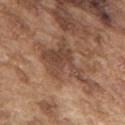The lesion was tiled from a total-body skin photograph and was not biopsied. The tile uses white-light illumination. From the back. The patient is a male roughly 75 years of age. Automated image analysis of the tile measured a lesion color around L≈45 a*≈19 b*≈28 in CIELAB, about 9 CIELAB-L* units darker than the surrounding skin, and a normalized border contrast of about 7. And it measured a border-irregularity rating of about 8.5/10, a within-lesion color-variation index near 4/10, and radial color variation of about 1.5. It also reported a classifier nevus-likeness of about 5/100 and a detector confidence of about 60 out of 100 that the crop contains a lesion. A region of skin cropped from a whole-body photographic capture, roughly 15 mm wide.